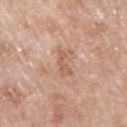| key | value |
|---|---|
| biopsy status | catalogued during a skin exam; not biopsied |
| patient | female, approximately 70 years of age |
| lighting | white-light |
| lesion diameter | about 3.5 mm |
| TBP lesion metrics | a footprint of about 3.5 mm² and a shape-asymmetry score of about 0.45 (0 = symmetric); border irregularity of about 5.5 on a 0–10 scale and internal color variation of about 0 on a 0–10 scale; a classifier nevus-likeness of about 0/100 and a lesion-detection confidence of about 100/100 |
| anatomic site | the right upper arm |
| image | 15 mm crop, total-body photography |